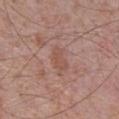Assessment: Recorded during total-body skin imaging; not selected for excision or biopsy. Background: Longest diameter approximately 3.5 mm. A close-up tile cropped from a whole-body skin photograph, about 15 mm across. Located on the chest. This is a white-light tile. The total-body-photography lesion software estimated an automated nevus-likeness rating near 0 out of 100 and lesion-presence confidence of about 100/100. The subject is a male aged approximately 70.